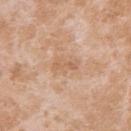Recorded during total-body skin imaging; not selected for excision or biopsy. On the right upper arm. A lesion tile, about 15 mm wide, cut from a 3D total-body photograph. A female subject aged 23 to 27. Imaged with white-light lighting. Measured at roughly 3 mm in maximum diameter.